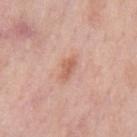Captured during whole-body skin photography for melanoma surveillance; the lesion was not biopsied. Located on the chest. A lesion tile, about 15 mm wide, cut from a 3D total-body photograph. The subject is a male roughly 55 years of age. The tile uses white-light illumination.